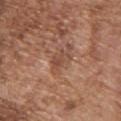workup: total-body-photography surveillance lesion; no biopsy
subject: male, aged 73 to 77
image: total-body-photography crop, ~15 mm field of view
lesion diameter: ~3 mm (longest diameter)
automated lesion analysis: a footprint of about 4.5 mm²; an average lesion color of about L≈48 a*≈21 b*≈29 (CIELAB) and a normalized lesion–skin contrast near 5.5; a nevus-likeness score of about 0/100
body site: the upper back